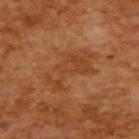The lesion was photographed on a routine skin check and not biopsied; there is no pathology result. A male subject, aged around 65. A 15 mm crop from a total-body photograph taken for skin-cancer surveillance.